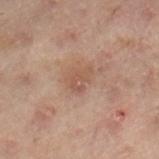Imaged during a routine full-body skin examination; the lesion was not biopsied and no histopathology is available. Cropped from a whole-body photographic skin survey; the tile spans about 15 mm. About 3 mm across. The patient is a female aged around 55. Imaged with cross-polarized lighting. The lesion is located on the left leg.